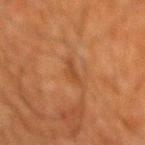The lesion was tiled from a total-body skin photograph and was not biopsied.
A roughly 15 mm field-of-view crop from a total-body skin photograph.
Imaged with cross-polarized lighting.
The lesion is on the back.
A male subject aged 78 to 82.
Approximately 3 mm at its widest.
The lesion-visualizer software estimated an area of roughly 3 mm² and a symmetry-axis asymmetry near 0.45. And it measured roughly 6 lightness units darker than nearby skin and a normalized border contrast of about 5.5. It also reported a nevus-likeness score of about 0/100.